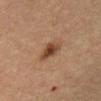Clinical impression:
This lesion was catalogued during total-body skin photography and was not selected for biopsy.
Background:
Cropped from a whole-body photographic skin survey; the tile spans about 15 mm. The lesion is located on the front of the torso. The lesion-visualizer software estimated an area of roughly 5.5 mm², a shape eccentricity near 0.85, and a shape-asymmetry score of about 0.3 (0 = symmetric). The analysis additionally found border irregularity of about 3 on a 0–10 scale, a color-variation rating of about 3/10, and radial color variation of about 0.5. The subject is a male aged 63–67. This is a cross-polarized tile.